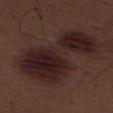Q: Was a biopsy performed?
A: imaged on a skin check; not biopsied